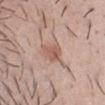biopsy status = catalogued during a skin exam; not biopsied | subject = male, aged 33–37 | imaging modality = total-body-photography crop, ~15 mm field of view | body site = the chest | diameter = ~3 mm (longest diameter).